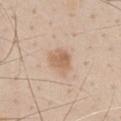This lesion was catalogued during total-body skin photography and was not selected for biopsy.
Longest diameter approximately 3 mm.
The subject is a male aged around 45.
From the abdomen.
Captured under white-light illumination.
Cropped from a total-body skin-imaging series; the visible field is about 15 mm.